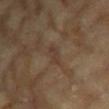Imaged during a routine full-body skin examination; the lesion was not biopsied and no histopathology is available.